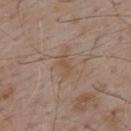Impression:
Captured during whole-body skin photography for melanoma surveillance; the lesion was not biopsied.
Background:
The tile uses white-light illumination. About 3 mm across. This image is a 15 mm lesion crop taken from a total-body photograph. The lesion is on the upper back. A male patient, aged approximately 55.